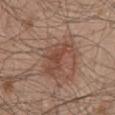Impression:
The lesion was photographed on a routine skin check and not biopsied; there is no pathology result.
Image and clinical context:
Captured under white-light illumination. A male subject aged 48 to 52. Automated tile analysis of the lesion measured a footprint of about 11 mm², a shape eccentricity near 0.8, and two-axis asymmetry of about 0.45. And it measured a lesion color around L≈46 a*≈20 b*≈27 in CIELAB, a lesion–skin lightness drop of about 8, and a normalized lesion–skin contrast near 6. And it measured a border-irregularity rating of about 6/10, internal color variation of about 3.5 on a 0–10 scale, and radial color variation of about 1. About 5 mm across. The lesion is located on the mid back. A lesion tile, about 15 mm wide, cut from a 3D total-body photograph.